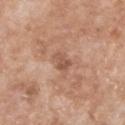Impression: The lesion was photographed on a routine skin check and not biopsied; there is no pathology result. Context: Cropped from a total-body skin-imaging series; the visible field is about 15 mm. From the leg. The subject is a male aged around 80.